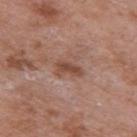No biopsy was performed on this lesion — it was imaged during a full skin examination and was not determined to be concerning.